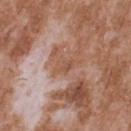| feature | finding |
|---|---|
| biopsy status | catalogued during a skin exam; not biopsied |
| patient | male, aged around 45 |
| image source | ~15 mm crop, total-body skin-cancer survey |
| body site | the back |
| diameter | ~2.5 mm (longest diameter) |
| illumination | white-light |
| automated metrics | a lesion area of about 3 mm², an eccentricity of roughly 0.8, and a symmetry-axis asymmetry near 0.3; border irregularity of about 3 on a 0–10 scale and radial color variation of about 0.5; an automated nevus-likeness rating near 0 out of 100 and a lesion-detection confidence of about 85/100 |